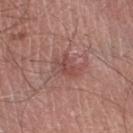notes: total-body-photography surveillance lesion; no biopsy | subject: male, in their mid-40s | site: the left lower leg | acquisition: 15 mm crop, total-body photography | lesion size: ≈3 mm.